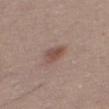Assessment: This lesion was catalogued during total-body skin photography and was not selected for biopsy. Acquisition and patient details: A roughly 15 mm field-of-view crop from a total-body skin photograph. The lesion-visualizer software estimated a mean CIELAB color near L≈49 a*≈19 b*≈24, roughly 9 lightness units darker than nearby skin, and a normalized border contrast of about 7. The lesion is on the right thigh. The subject is a female roughly 50 years of age. Imaged with white-light lighting. Approximately 3 mm at its widest.